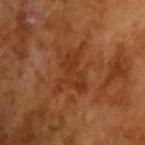<record>
<lighting>cross-polarized</lighting>
<lesion_size>
  <long_diameter_mm_approx>5.0</long_diameter_mm_approx>
</lesion_size>
<patient>
  <sex>male</sex>
  <age_approx>65</age_approx>
</patient>
<image>
  <source>total-body photography crop</source>
  <field_of_view_mm>15</field_of_view_mm>
</image>
</record>